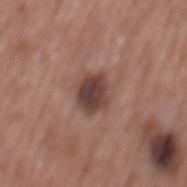{
  "biopsy_status": "not biopsied; imaged during a skin examination",
  "site": "mid back",
  "patient": {
    "sex": "male",
    "age_approx": 75
  },
  "automated_metrics": {
    "cielab_L": 42,
    "cielab_a": 19,
    "cielab_b": 21,
    "vs_skin_darker_L": 13.0,
    "vs_skin_contrast_norm": 10.0,
    "nevus_likeness_0_100": 20,
    "lesion_detection_confidence_0_100": 100
  },
  "lighting": "white-light",
  "lesion_size": {
    "long_diameter_mm_approx": 3.5
  },
  "image": {
    "source": "total-body photography crop",
    "field_of_view_mm": 15
  }
}